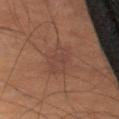follow-up — catalogued during a skin exam; not biopsied
patient — male, aged approximately 70
imaging modality — ~15 mm tile from a whole-body skin photo
lighting — cross-polarized
anatomic site — the left thigh
TBP lesion metrics — a lesion area of about 8.5 mm², an outline eccentricity of about 0.75 (0 = round, 1 = elongated), and two-axis asymmetry of about 0.25; a lesion color around L≈31 a*≈15 b*≈20 in CIELAB, about 4 CIELAB-L* units darker than the surrounding skin, and a lesion-to-skin contrast of about 4.5 (normalized; higher = more distinct); a border-irregularity index near 2.5/10, a color-variation rating of about 2/10, and peripheral color asymmetry of about 0.5; a classifier nevus-likeness of about 0/100 and lesion-presence confidence of about 100/100
lesion size — ~4 mm (longest diameter)